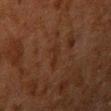{
  "biopsy_status": "not biopsied; imaged during a skin examination",
  "patient": {
    "sex": "male",
    "age_approx": 60
  },
  "image": {
    "source": "total-body photography crop",
    "field_of_view_mm": 15
  },
  "lesion_size": {
    "long_diameter_mm_approx": 3.0
  },
  "lighting": "cross-polarized",
  "site": "right upper arm"
}